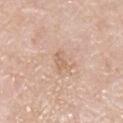biopsy status=no biopsy performed (imaged during a skin exam)